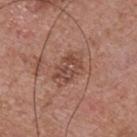Assessment: This lesion was catalogued during total-body skin photography and was not selected for biopsy. Context: On the chest. This is a white-light tile. The lesion-visualizer software estimated a symmetry-axis asymmetry near 0.3. The software also gave a mean CIELAB color near L≈46 a*≈22 b*≈26, about 9 CIELAB-L* units darker than the surrounding skin, and a normalized border contrast of about 6.5. The analysis additionally found a nevus-likeness score of about 0/100 and lesion-presence confidence of about 100/100. Measured at roughly 3.5 mm in maximum diameter. A 15 mm close-up tile from a total-body photography series done for melanoma screening. The patient is a male aged 53 to 57.